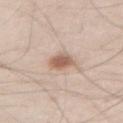Part of a total-body skin-imaging series; this lesion was reviewed on a skin check and was not flagged for biopsy. A male patient aged 63–67. Cropped from a whole-body photographic skin survey; the tile spans about 15 mm. From the abdomen. Automated tile analysis of the lesion measured an outline eccentricity of about 0.7 (0 = round, 1 = elongated) and two-axis asymmetry of about 0.15. It also reported an average lesion color of about L≈60 a*≈18 b*≈28 (CIELAB), roughly 13 lightness units darker than nearby skin, and a normalized border contrast of about 8.5. The software also gave a within-lesion color-variation index near 4/10 and peripheral color asymmetry of about 1.5. The analysis additionally found a classifier nevus-likeness of about 95/100 and a lesion-detection confidence of about 100/100. About 3 mm across.